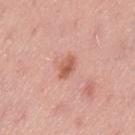{"biopsy_status": "not biopsied; imaged during a skin examination", "patient": {"sex": "male", "age_approx": 50}, "site": "left thigh", "image": {"source": "total-body photography crop", "field_of_view_mm": 15}, "automated_metrics": {"eccentricity": 0.8, "shape_asymmetry": 0.2, "border_irregularity_0_10": 2.0, "color_variation_0_10": 2.5, "peripheral_color_asymmetry": 1.0}}